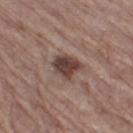{
  "biopsy_status": "not biopsied; imaged during a skin examination",
  "image": {
    "source": "total-body photography crop",
    "field_of_view_mm": 15
  },
  "site": "right thigh",
  "patient": {
    "sex": "male",
    "age_approx": 65
  },
  "lesion_size": {
    "long_diameter_mm_approx": 3.5
  }
}